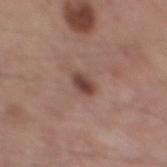The lesion was photographed on a routine skin check and not biopsied; there is no pathology result. A male subject in their mid-50s. A 15 mm close-up extracted from a 3D total-body photography capture. An algorithmic analysis of the crop reported a lesion area of about 3.5 mm² and an eccentricity of roughly 0.75. It also reported a mean CIELAB color near L≈43 a*≈20 b*≈23 and roughly 12 lightness units darker than nearby skin. The analysis additionally found a border-irregularity rating of about 1.5/10, a color-variation rating of about 3/10, and peripheral color asymmetry of about 1. Measured at roughly 2.5 mm in maximum diameter. The lesion is on the mid back. Captured under white-light illumination.